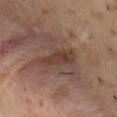• workup — total-body-photography surveillance lesion; no biopsy